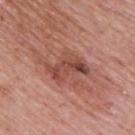biopsy status = catalogued during a skin exam; not biopsied | body site = the back | size = about 5 mm | automated metrics = a lesion color around L≈47 a*≈25 b*≈27 in CIELAB, roughly 10 lightness units darker than nearby skin, and a normalized lesion–skin contrast near 7.5; a border-irregularity index near 5.5/10 | patient = male, aged 73 to 77 | lighting = white-light | imaging modality = ~15 mm crop, total-body skin-cancer survey.